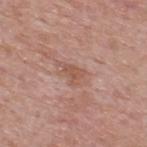No biopsy was performed on this lesion — it was imaged during a full skin examination and was not determined to be concerning.